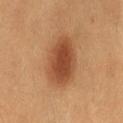Recorded during total-body skin imaging; not selected for excision or biopsy. A female patient approximately 40 years of age. The lesion is located on the left thigh. The recorded lesion diameter is about 6 mm. A roughly 15 mm field-of-view crop from a total-body skin photograph. This is a cross-polarized tile. The total-body-photography lesion software estimated a lesion color around L≈47 a*≈24 b*≈35 in CIELAB, a lesion–skin lightness drop of about 13, and a lesion-to-skin contrast of about 9 (normalized; higher = more distinct).